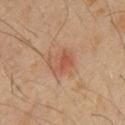Assessment:
The lesion was tiled from a total-body skin photograph and was not biopsied.
Context:
On the front of the torso. About 3.5 mm across. A male patient approximately 60 years of age. Imaged with cross-polarized lighting. The total-body-photography lesion software estimated a footprint of about 6 mm², an outline eccentricity of about 0.7 (0 = round, 1 = elongated), and a symmetry-axis asymmetry near 0.4. And it measured roughly 7 lightness units darker than nearby skin. It also reported a classifier nevus-likeness of about 25/100 and lesion-presence confidence of about 100/100. Cropped from a whole-body photographic skin survey; the tile spans about 15 mm.